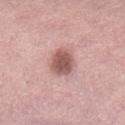Clinical impression: Part of a total-body skin-imaging series; this lesion was reviewed on a skin check and was not flagged for biopsy. Clinical summary: Located on the lower back. A roughly 15 mm field-of-view crop from a total-body skin photograph. Approximately 3.5 mm at its widest. This is a white-light tile. A female subject aged 63 to 67.